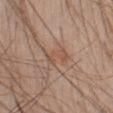Recorded during total-body skin imaging; not selected for excision or biopsy.
A male subject approximately 40 years of age.
A region of skin cropped from a whole-body photographic capture, roughly 15 mm wide.
Located on the abdomen.
The tile uses white-light illumination.
The recorded lesion diameter is about 3.5 mm.